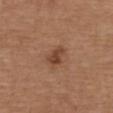Captured during whole-body skin photography for melanoma surveillance; the lesion was not biopsied.
From the back.
A region of skin cropped from a whole-body photographic capture, roughly 15 mm wide.
Imaged with white-light lighting.
A female subject, roughly 40 years of age.